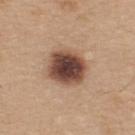{
  "biopsy_status": "not biopsied; imaged during a skin examination",
  "patient": {
    "sex": "male",
    "age_approx": 35
  },
  "site": "upper back",
  "image": {
    "source": "total-body photography crop",
    "field_of_view_mm": 15
  }
}